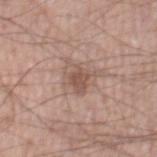Assessment:
This lesion was catalogued during total-body skin photography and was not selected for biopsy.
Acquisition and patient details:
Longest diameter approximately 3 mm. The tile uses white-light illumination. A 15 mm close-up tile from a total-body photography series done for melanoma screening. A male subject, aged 58–62. Located on the right thigh.